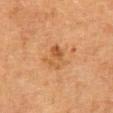notes: catalogued during a skin exam; not biopsied | automated lesion analysis: an area of roughly 5 mm² and an eccentricity of roughly 0.75; an average lesion color of about L≈45 a*≈21 b*≈35 (CIELAB) and a lesion-to-skin contrast of about 6 (normalized; higher = more distinct) | size: ≈3 mm | patient: female, in their 50s | tile lighting: cross-polarized | anatomic site: the abdomen | image source: ~15 mm tile from a whole-body skin photo.